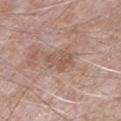Q: Is there a histopathology result?
A: imaged on a skin check; not biopsied
Q: Illumination type?
A: white-light illumination
Q: What is the imaging modality?
A: 15 mm crop, total-body photography
Q: What is the lesion's diameter?
A: about 3.5 mm
Q: What are the patient's age and sex?
A: male, about 75 years old
Q: What did automated image analysis measure?
A: border irregularity of about 4.5 on a 0–10 scale, internal color variation of about 2 on a 0–10 scale, and a peripheral color-asymmetry measure near 0.5; an automated nevus-likeness rating near 0 out of 100 and a detector confidence of about 100 out of 100 that the crop contains a lesion
Q: Lesion location?
A: the leg